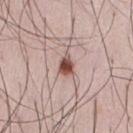Q: Is there a histopathology result?
A: imaged on a skin check; not biopsied
Q: What did automated image analysis measure?
A: radial color variation of about 1.5
Q: Lesion location?
A: the chest
Q: Patient demographics?
A: male, in their mid-30s
Q: How was the tile lit?
A: white-light illumination
Q: What is the imaging modality?
A: ~15 mm crop, total-body skin-cancer survey